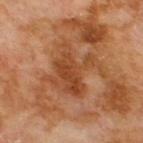The lesion was photographed on a routine skin check and not biopsied; there is no pathology result. This image is a 15 mm lesion crop taken from a total-body photograph. A male subject aged 68–72. The lesion-visualizer software estimated a lesion–skin lightness drop of about 8 and a normalized border contrast of about 6.5. And it measured border irregularity of about 8.5 on a 0–10 scale, a color-variation rating of about 5/10, and peripheral color asymmetry of about 1.5. Located on the upper back.